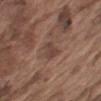Q: Is there a histopathology result?
A: catalogued during a skin exam; not biopsied
Q: Illumination type?
A: white-light illumination
Q: How large is the lesion?
A: ≈3.5 mm
Q: What are the patient's age and sex?
A: male, in their mid-70s
Q: Where on the body is the lesion?
A: the left upper arm
Q: How was this image acquired?
A: total-body-photography crop, ~15 mm field of view